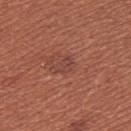notes=catalogued during a skin exam; not biopsied | lighting=white-light illumination | site=the chest | lesion size=about 2.5 mm | subject=female, approximately 35 years of age | image source=total-body-photography crop, ~15 mm field of view | image-analysis metrics=an area of roughly 3.5 mm², a shape eccentricity near 0.8, and two-axis asymmetry of about 0.25; a lesion–skin lightness drop of about 6 and a normalized border contrast of about 5.5; a nevus-likeness score of about 10/100.